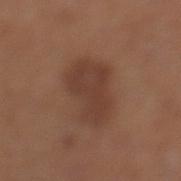Q: Was this lesion biopsied?
A: imaged on a skin check; not biopsied
Q: What kind of image is this?
A: total-body-photography crop, ~15 mm field of view
Q: Lesion location?
A: the left lower leg
Q: What are the patient's age and sex?
A: female, in their 40s
Q: Illumination type?
A: white-light